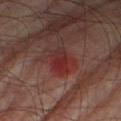Clinical impression:
The lesion was tiled from a total-body skin photograph and was not biopsied.
Image and clinical context:
Automated image analysis of the tile measured a footprint of about 4.5 mm², an eccentricity of roughly 0.8, and a shape-asymmetry score of about 0.2 (0 = symmetric). The analysis additionally found an average lesion color of about L≈24 a*≈24 b*≈19 (CIELAB). The software also gave an automated nevus-likeness rating near 50 out of 100 and a detector confidence of about 100 out of 100 that the crop contains a lesion. A male subject approximately 75 years of age. On the right thigh. A 15 mm close-up tile from a total-body photography series done for melanoma screening. Captured under cross-polarized illumination.